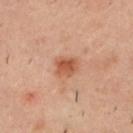biopsy_status: not biopsied; imaged during a skin examination
site: upper back
automated_metrics:
  nevus_likeness_0_100: 90
lighting: cross-polarized
patient:
  sex: male
  age_approx: 40
image:
  source: total-body photography crop
  field_of_view_mm: 15
lesion_size:
  long_diameter_mm_approx: 3.0